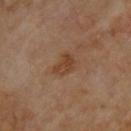{"biopsy_status": "not biopsied; imaged during a skin examination", "patient": {"sex": "male", "age_approx": 70}, "site": "upper back", "lighting": "cross-polarized", "image": {"source": "total-body photography crop", "field_of_view_mm": 15}, "automated_metrics": {"eccentricity": 0.75, "shape_asymmetry": 0.35, "cielab_L": 40, "cielab_a": 19, "cielab_b": 31, "vs_skin_darker_L": 7.0, "vs_skin_contrast_norm": 7.0}, "lesion_size": {"long_diameter_mm_approx": 3.0}}